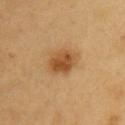| feature | finding |
|---|---|
| follow-up | total-body-photography surveillance lesion; no biopsy |
| lesion diameter | about 4 mm |
| automated metrics | a lesion area of about 9.5 mm², an eccentricity of roughly 0.65, and a shape-asymmetry score of about 0.15 (0 = symmetric); border irregularity of about 1.5 on a 0–10 scale and internal color variation of about 5.5 on a 0–10 scale |
| site | the chest |
| lighting | cross-polarized |
| patient | male, roughly 60 years of age |
| image source | total-body-photography crop, ~15 mm field of view |